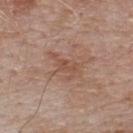biopsy_status: not biopsied; imaged during a skin examination
patient:
  sex: male
  age_approx: 55
lighting: white-light
image:
  source: total-body photography crop
  field_of_view_mm: 15
site: upper back
automated_metrics:
  area_mm2_approx: 5.5
  eccentricity: 0.85
  shape_asymmetry: 0.5
  border_irregularity_0_10: 6.5
  color_variation_0_10: 2.0